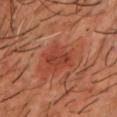Q: Was a biopsy performed?
A: total-body-photography surveillance lesion; no biopsy
Q: What is the anatomic site?
A: the chest
Q: What lighting was used for the tile?
A: cross-polarized
Q: What did automated image analysis measure?
A: an area of roughly 6.5 mm², an outline eccentricity of about 0.7 (0 = round, 1 = elongated), and a shape-asymmetry score of about 0.35 (0 = symmetric); a lesion color around L≈42 a*≈31 b*≈34 in CIELAB, roughly 8 lightness units darker than nearby skin, and a lesion-to-skin contrast of about 6 (normalized; higher = more distinct); border irregularity of about 3.5 on a 0–10 scale, internal color variation of about 2.5 on a 0–10 scale, and peripheral color asymmetry of about 1; a nevus-likeness score of about 35/100 and lesion-presence confidence of about 100/100
Q: Who is the patient?
A: male, aged 38 to 42
Q: What is the imaging modality?
A: ~15 mm crop, total-body skin-cancer survey
Q: Lesion size?
A: ≈3.5 mm